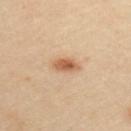Assessment:
Recorded during total-body skin imaging; not selected for excision or biopsy.
Acquisition and patient details:
The recorded lesion diameter is about 3 mm. A male patient, about 35 years old. Cropped from a total-body skin-imaging series; the visible field is about 15 mm. The lesion is located on the upper back. Captured under cross-polarized illumination.